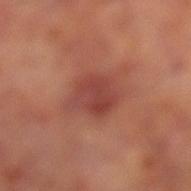  biopsy_status: not biopsied; imaged during a skin examination
  lighting: cross-polarized
  patient:
    sex: male
    age_approx: 65
  site: left lower leg
  image:
    source: total-body photography crop
    field_of_view_mm: 15
  lesion_size:
    long_diameter_mm_approx: 5.5